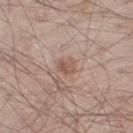Clinical impression: The lesion was photographed on a routine skin check and not biopsied; there is no pathology result. Background: The total-body-photography lesion software estimated a lesion color around L≈55 a*≈18 b*≈26 in CIELAB and about 8 CIELAB-L* units darker than the surrounding skin. The subject is a male aged 58–62. The lesion is on the right thigh. The tile uses white-light illumination. Cropped from a total-body skin-imaging series; the visible field is about 15 mm.